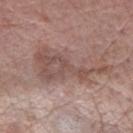workup: imaged on a skin check; not biopsied | location: the right forearm | subject: female, aged 68–72 | automated metrics: an area of roughly 24 mm²; a mean CIELAB color near L≈51 a*≈18 b*≈22, roughly 9 lightness units darker than nearby skin, and a lesion-to-skin contrast of about 6.5 (normalized; higher = more distinct); a color-variation rating of about 4/10 and peripheral color asymmetry of about 1; a lesion-detection confidence of about 55/100 | diameter: about 9.5 mm | imaging modality: ~15 mm crop, total-body skin-cancer survey.